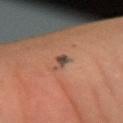Captured during whole-body skin photography for melanoma surveillance; the lesion was not biopsied.
A male subject, in their 40s.
Imaged with cross-polarized lighting.
The lesion is on the left forearm.
The total-body-photography lesion software estimated a peripheral color-asymmetry measure near 0.5.
A roughly 15 mm field-of-view crop from a total-body skin photograph.